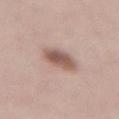{
  "patient": {
    "sex": "female",
    "age_approx": 55
  },
  "automated_metrics": {
    "nevus_likeness_0_100": 90,
    "lesion_detection_confidence_0_100": 100
  },
  "site": "lower back",
  "image": {
    "source": "total-body photography crop",
    "field_of_view_mm": 15
  }
}